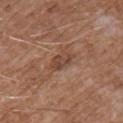Q: Is there a histopathology result?
A: catalogued during a skin exam; not biopsied
Q: Illumination type?
A: white-light illumination
Q: Where on the body is the lesion?
A: the upper back
Q: Who is the patient?
A: male, roughly 60 years of age
Q: How was this image acquired?
A: total-body-photography crop, ~15 mm field of view
Q: What is the lesion's diameter?
A: about 3.5 mm